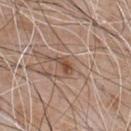Clinical impression: The lesion was photographed on a routine skin check and not biopsied; there is no pathology result. Context: This is a white-light tile. Cropped from a total-body skin-imaging series; the visible field is about 15 mm. Located on the chest. A male patient, in their mid-60s.